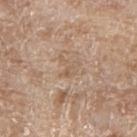Assessment: This lesion was catalogued during total-body skin photography and was not selected for biopsy.